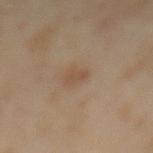Assessment: Captured during whole-body skin photography for melanoma surveillance; the lesion was not biopsied. Background: Captured under cross-polarized illumination. Longest diameter approximately 2.5 mm. A region of skin cropped from a whole-body photographic capture, roughly 15 mm wide. The patient is a female roughly 55 years of age. From the mid back. Automated image analysis of the tile measured a border-irregularity rating of about 3/10, a within-lesion color-variation index near 0.5/10, and radial color variation of about 0. The software also gave a classifier nevus-likeness of about 5/100 and a detector confidence of about 100 out of 100 that the crop contains a lesion.